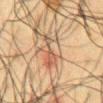follow-up=catalogued during a skin exam; not biopsied
patient=male, aged 58–62
anatomic site=the chest
lighting=cross-polarized illumination
image=~15 mm crop, total-body skin-cancer survey
lesion diameter=~4.5 mm (longest diameter)